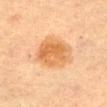Imaged during a routine full-body skin examination; the lesion was not biopsied and no histopathology is available.